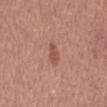Part of a total-body skin-imaging series; this lesion was reviewed on a skin check and was not flagged for biopsy. A roughly 15 mm field-of-view crop from a total-body skin photograph. Located on the mid back. The subject is a male in their mid-60s.